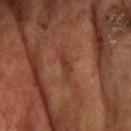follow-up — no biopsy performed (imaged during a skin exam) | imaging modality — ~15 mm crop, total-body skin-cancer survey | lesion size — ≈3.5 mm | body site — the head or neck | automated metrics — a mean CIELAB color near L≈35 a*≈25 b*≈30, about 7 CIELAB-L* units darker than the surrounding skin, and a normalized lesion–skin contrast near 6.5; a border-irregularity rating of about 6/10, a color-variation rating of about 0/10, and radial color variation of about 0 | lighting — cross-polarized | patient — male, approximately 70 years of age.